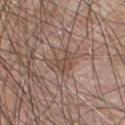Impression:
Captured during whole-body skin photography for melanoma surveillance; the lesion was not biopsied.
Clinical summary:
Approximately 3.5 mm at its widest. Cropped from a total-body skin-imaging series; the visible field is about 15 mm. Captured under white-light illumination. The subject is a male roughly 60 years of age. The lesion is located on the chest. Automated image analysis of the tile measured a mean CIELAB color near L≈49 a*≈16 b*≈25, a lesion–skin lightness drop of about 7, and a lesion-to-skin contrast of about 6 (normalized; higher = more distinct). The software also gave border irregularity of about 6 on a 0–10 scale, internal color variation of about 2.5 on a 0–10 scale, and radial color variation of about 1.